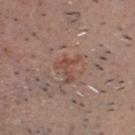Imaged during a routine full-body skin examination; the lesion was not biopsied and no histopathology is available. Located on the head or neck. Captured under white-light illumination. A 15 mm crop from a total-body photograph taken for skin-cancer surveillance. A male patient about 50 years old.